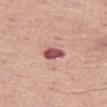{"biopsy_status": "not biopsied; imaged during a skin examination", "patient": {"sex": "male", "age_approx": 75}, "site": "left thigh", "lesion_size": {"long_diameter_mm_approx": 2.5}, "lighting": "white-light", "image": {"source": "total-body photography crop", "field_of_view_mm": 15}}